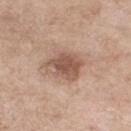Acquisition and patient details: Captured under white-light illumination. The lesion-visualizer software estimated a footprint of about 10 mm², an outline eccentricity of about 0.65 (0 = round, 1 = elongated), and a symmetry-axis asymmetry near 0.2. The software also gave border irregularity of about 2 on a 0–10 scale and internal color variation of about 4 on a 0–10 scale. And it measured a nevus-likeness score of about 40/100 and a detector confidence of about 100 out of 100 that the crop contains a lesion. A 15 mm close-up extracted from a 3D total-body photography capture. A female subject approximately 55 years of age. On the right thigh.